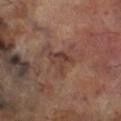– notes — catalogued during a skin exam; not biopsied
– image — ~15 mm crop, total-body skin-cancer survey
– subject — male, aged around 70
– TBP lesion metrics — a lesion area of about 4.5 mm², an eccentricity of roughly 0.6, and a symmetry-axis asymmetry near 0.65; a mean CIELAB color near L≈37 a*≈19 b*≈24, about 7 CIELAB-L* units darker than the surrounding skin, and a normalized border contrast of about 6
– location — the leg
– lesion size — ~3 mm (longest diameter)
– lighting — cross-polarized